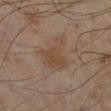Findings:
– workup: total-body-photography surveillance lesion; no biopsy
– TBP lesion metrics: internal color variation of about 2 on a 0–10 scale and radial color variation of about 0.5; a lesion-detection confidence of about 100/100
– lesion size: ~4.5 mm (longest diameter)
– location: the left lower leg
– tile lighting: cross-polarized illumination
– patient: male, roughly 45 years of age
– image source: ~15 mm crop, total-body skin-cancer survey